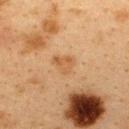notes — catalogued during a skin exam; not biopsied
patient — female, roughly 40 years of age
image source — ~15 mm tile from a whole-body skin photo
tile lighting — cross-polarized
body site — the upper back
size — about 3 mm